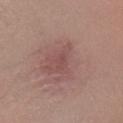No biopsy was performed on this lesion — it was imaged during a full skin examination and was not determined to be concerning. From the right lower leg. A 15 mm crop from a total-body photograph taken for skin-cancer surveillance. This is a white-light tile. The subject is a female aged around 55.